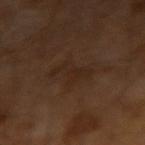Assessment: The lesion was tiled from a total-body skin photograph and was not biopsied. Background: Measured at roughly 4 mm in maximum diameter. Imaged with cross-polarized lighting. A close-up tile cropped from a whole-body skin photograph, about 15 mm across. A male patient aged approximately 65.